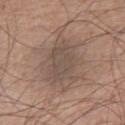  biopsy_status: not biopsied; imaged during a skin examination
  site: right thigh
  patient:
    sex: male
    age_approx: 60
  image:
    source: total-body photography crop
    field_of_view_mm: 15